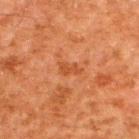Clinical impression:
Recorded during total-body skin imaging; not selected for excision or biopsy.
Image and clinical context:
The lesion is located on the upper back. The total-body-photography lesion software estimated an area of roughly 2.5 mm², a shape eccentricity near 0.9, and a symmetry-axis asymmetry near 0.5. It also reported a mean CIELAB color near L≈39 a*≈25 b*≈35. Measured at roughly 2.5 mm in maximum diameter. A 15 mm crop from a total-body photograph taken for skin-cancer surveillance. A male subject aged around 60. The tile uses cross-polarized illumination.